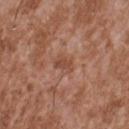biopsy status: imaged on a skin check; not biopsied
lighting: white-light illumination
body site: the upper back
image source: total-body-photography crop, ~15 mm field of view
subject: male, in their mid- to late 40s